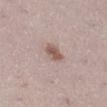Impression: The lesion was photographed on a routine skin check and not biopsied; there is no pathology result.